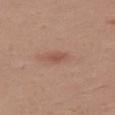No biopsy was performed on this lesion — it was imaged during a full skin examination and was not determined to be concerning.
The lesion is located on the leg.
The subject is a female in their 30s.
A 15 mm crop from a total-body photograph taken for skin-cancer surveillance.
The recorded lesion diameter is about 2.5 mm.
Captured under white-light illumination.